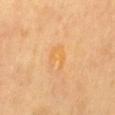Recorded during total-body skin imaging; not selected for excision or biopsy. The lesion is located on the back. The tile uses cross-polarized illumination. A 15 mm close-up tile from a total-body photography series done for melanoma screening. The recorded lesion diameter is about 4 mm. The total-body-photography lesion software estimated an average lesion color of about L≈68 a*≈22 b*≈47 (CIELAB) and a normalized lesion–skin contrast near 5.5. It also reported a border-irregularity rating of about 6.5/10, internal color variation of about 2.5 on a 0–10 scale, and a peripheral color-asymmetry measure near 1. And it measured a nevus-likeness score of about 0/100 and lesion-presence confidence of about 100/100. A male subject, about 60 years old.